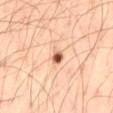• biopsy status: total-body-photography surveillance lesion; no biopsy
• anatomic site: the leg
• imaging modality: ~15 mm crop, total-body skin-cancer survey
• diameter: ≈4.5 mm
• tile lighting: cross-polarized illumination
• patient: male, about 45 years old
• TBP lesion metrics: a lesion area of about 7 mm²; a mean CIELAB color near L≈66 a*≈20 b*≈32 and about 10 CIELAB-L* units darker than the surrounding skin; a classifier nevus-likeness of about 95/100 and lesion-presence confidence of about 100/100